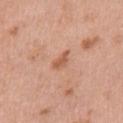Impression: Captured during whole-body skin photography for melanoma surveillance; the lesion was not biopsied. Clinical summary: The lesion is on the chest. Automated image analysis of the tile measured an eccentricity of roughly 0.85 and a shape-asymmetry score of about 0.4 (0 = symmetric). And it measured an automated nevus-likeness rating near 50 out of 100 and lesion-presence confidence of about 100/100. The tile uses white-light illumination. Longest diameter approximately 2.5 mm. This image is a 15 mm lesion crop taken from a total-body photograph. The subject is a female about 40 years old.